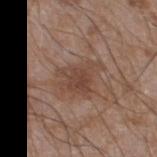Part of a total-body skin-imaging series; this lesion was reviewed on a skin check and was not flagged for biopsy. Measured at roughly 5 mm in maximum diameter. The tile uses white-light illumination. From the left thigh. A male patient roughly 60 years of age. A 15 mm close-up tile from a total-body photography series done for melanoma screening.